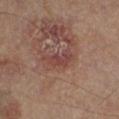• automated lesion analysis · an eccentricity of roughly 0.85; an average lesion color of about L≈40 a*≈22 b*≈22 (CIELAB), a lesion–skin lightness drop of about 7, and a lesion-to-skin contrast of about 6.5 (normalized; higher = more distinct); a border-irregularity rating of about 4.5/10, internal color variation of about 2.5 on a 0–10 scale, and a peripheral color-asymmetry measure near 1; lesion-presence confidence of about 100/100
• image · total-body-photography crop, ~15 mm field of view
• subject · male, aged approximately 65
• location · the left lower leg
• tile lighting · cross-polarized illumination
• lesion size · ≈4 mm